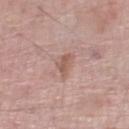follow-up: no biopsy performed (imaged during a skin exam)
body site: the right lower leg
image source: ~15 mm crop, total-body skin-cancer survey
patient: male, roughly 70 years of age
TBP lesion metrics: a lesion area of about 3 mm² and a shape-asymmetry score of about 0.45 (0 = symmetric); a mean CIELAB color near L≈56 a*≈20 b*≈26, a lesion–skin lightness drop of about 9, and a normalized lesion–skin contrast near 6.5; a detector confidence of about 100 out of 100 that the crop contains a lesion